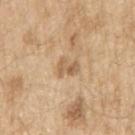Recorded during total-body skin imaging; not selected for excision or biopsy. On the right upper arm. A 15 mm crop from a total-body photograph taken for skin-cancer surveillance. Longest diameter approximately 2.5 mm. A male patient aged approximately 70. This is a white-light tile. An algorithmic analysis of the crop reported two-axis asymmetry of about 0.35. The software also gave an average lesion color of about L≈60 a*≈17 b*≈35 (CIELAB) and a normalized lesion–skin contrast near 6.5. The software also gave a border-irregularity index near 4/10, internal color variation of about 4 on a 0–10 scale, and peripheral color asymmetry of about 1. The software also gave a classifier nevus-likeness of about 0/100 and a detector confidence of about 100 out of 100 that the crop contains a lesion.